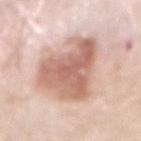No biopsy was performed on this lesion — it was imaged during a full skin examination and was not determined to be concerning. This image is a 15 mm lesion crop taken from a total-body photograph. The lesion's longest dimension is about 8 mm. Captured under white-light illumination. Located on the abdomen. The lesion-visualizer software estimated an average lesion color of about L≈64 a*≈21 b*≈27 (CIELAB) and a lesion-to-skin contrast of about 8.5 (normalized; higher = more distinct). It also reported border irregularity of about 4.5 on a 0–10 scale and a peripheral color-asymmetry measure near 2. The analysis additionally found an automated nevus-likeness rating near 85 out of 100. The subject is a male aged 78 to 82.